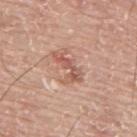workup: no biopsy performed (imaged during a skin exam) | image-analysis metrics: a lesion area of about 6.5 mm², an outline eccentricity of about 0.9 (0 = round, 1 = elongated), and a symmetry-axis asymmetry near 0.45 | illumination: white-light illumination | patient: male, in their mid- to late 70s | imaging modality: total-body-photography crop, ~15 mm field of view | site: the upper back.